On the chest · a female subject, aged 38–42 · a region of skin cropped from a whole-body photographic capture, roughly 15 mm wide:
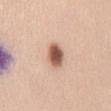illumination: white-light illumination
diameter: ≈3.5 mm
histopathology: a dysplastic (Clark) nevus